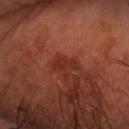Located on the right forearm. Imaged with cross-polarized lighting. A male patient aged 68 to 72. A region of skin cropped from a whole-body photographic capture, roughly 15 mm wide.